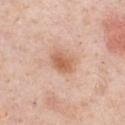body site: the chest
subject: male, about 50 years old
tile lighting: white-light
lesion size: ~3.5 mm (longest diameter)
acquisition: ~15 mm crop, total-body skin-cancer survey